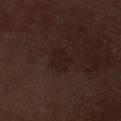This lesion was catalogued during total-body skin photography and was not selected for biopsy. This is a white-light tile. On the left lower leg. Longest diameter approximately 3.5 mm. A male patient aged approximately 70. A roughly 15 mm field-of-view crop from a total-body skin photograph. An algorithmic analysis of the crop reported a border-irregularity index near 2.5/10 and radial color variation of about 0.5. The software also gave an automated nevus-likeness rating near 0 out of 100 and lesion-presence confidence of about 100/100.